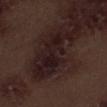Assessment: The lesion was tiled from a total-body skin photograph and was not biopsied. Acquisition and patient details: This is a white-light tile. A 15 mm crop from a total-body photograph taken for skin-cancer surveillance. A male subject, about 70 years old. The recorded lesion diameter is about 9 mm. The lesion is on the leg.